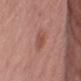No biopsy was performed on this lesion — it was imaged during a full skin examination and was not determined to be concerning. From the left upper arm. A male patient aged 53 to 57. This image is a 15 mm lesion crop taken from a total-body photograph. The tile uses white-light illumination. The lesion's longest dimension is about 3 mm.